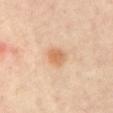{
  "lighting": "cross-polarized",
  "image": {
    "source": "total-body photography crop",
    "field_of_view_mm": 15
  },
  "patient": {
    "sex": "male",
    "age_approx": 55
  },
  "site": "abdomen"
}